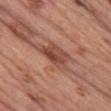A 15 mm crop from a total-body photograph taken for skin-cancer surveillance.
This is a white-light tile.
The patient is a female approximately 60 years of age.
The lesion is located on the chest.